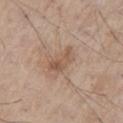notes: catalogued during a skin exam; not biopsied | tile lighting: white-light | acquisition: ~15 mm crop, total-body skin-cancer survey | TBP lesion metrics: a mean CIELAB color near L≈55 a*≈17 b*≈29, roughly 8 lightness units darker than nearby skin, and a normalized lesion–skin contrast near 6 | patient: male, aged around 80 | size: ~4 mm (longest diameter) | site: the chest.